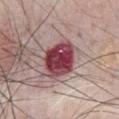Part of a total-body skin-imaging series; this lesion was reviewed on a skin check and was not flagged for biopsy. The lesion's longest dimension is about 5 mm. The patient is a male approximately 70 years of age. From the chest. A 15 mm close-up extracted from a 3D total-body photography capture. This is a white-light tile.